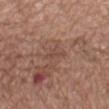The patient is a female in their mid-70s. Cropped from a whole-body photographic skin survey; the tile spans about 15 mm. The lesion's longest dimension is about 3 mm. The lesion is located on the mid back. This is a white-light tile.